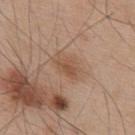follow-up = imaged on a skin check; not biopsied | subject = male, aged 53 to 57 | lesion diameter = ~3 mm (longest diameter) | illumination = white-light illumination | image = ~15 mm crop, total-body skin-cancer survey | site = the upper back.